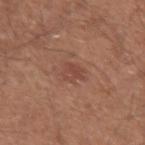This lesion was catalogued during total-body skin photography and was not selected for biopsy. Approximately 2.5 mm at its widest. A male subject, approximately 55 years of age. The lesion is on the left upper arm. The tile uses white-light illumination. Cropped from a total-body skin-imaging series; the visible field is about 15 mm.